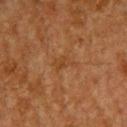workup: total-body-photography surveillance lesion; no biopsy | image: 15 mm crop, total-body photography | size: ~3 mm (longest diameter) | illumination: cross-polarized | location: the upper back | automated lesion analysis: a border-irregularity rating of about 4.5/10 and a within-lesion color-variation index near 1/10 | patient: male, about 60 years old.